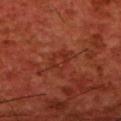<tbp_lesion>
<biopsy_status>not biopsied; imaged during a skin examination</biopsy_status>
<patient>
  <sex>male</sex>
  <age_approx>60</age_approx>
</patient>
<site>back</site>
<image>
  <source>total-body photography crop</source>
  <field_of_view_mm>15</field_of_view_mm>
</image>
</tbp_lesion>